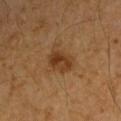The lesion was photographed on a routine skin check and not biopsied; there is no pathology result. A male patient in their 60s. A 15 mm close-up extracted from a 3D total-body photography capture. The lesion is on the right upper arm.